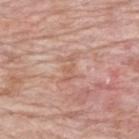workup = no biopsy performed (imaged during a skin exam) | imaging modality = ~15 mm crop, total-body skin-cancer survey | location = the mid back | lighting = white-light | automated lesion analysis = a shape eccentricity near 0.9 and a symmetry-axis asymmetry near 0.4; a lesion color around L≈60 a*≈22 b*≈29 in CIELAB and roughly 7 lightness units darker than nearby skin; a border-irregularity rating of about 6/10, internal color variation of about 0 on a 0–10 scale, and radial color variation of about 0; an automated nevus-likeness rating near 0 out of 100 | subject = male, approximately 60 years of age | lesion diameter = ≈3 mm.